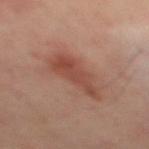No biopsy was performed on this lesion — it was imaged during a full skin examination and was not determined to be concerning.
From the mid back.
Automated tile analysis of the lesion measured a shape-asymmetry score of about 0.35 (0 = symmetric). The software also gave a lesion color around L≈46 a*≈24 b*≈28 in CIELAB, about 9 CIELAB-L* units darker than the surrounding skin, and a normalized border contrast of about 7.
A male patient aged 48 to 52.
A roughly 15 mm field-of-view crop from a total-body skin photograph.
The lesion's longest dimension is about 6 mm.